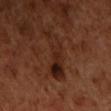biopsy status = no biopsy performed (imaged during a skin exam)
imaging modality = 15 mm crop, total-body photography
size = ~6.5 mm (longest diameter)
patient = male, about 50 years old
site = the chest
lighting = cross-polarized